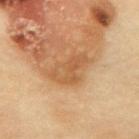This lesion was catalogued during total-body skin photography and was not selected for biopsy. On the upper back. A female subject aged 58 to 62. A 15 mm close-up extracted from a 3D total-body photography capture. Automated tile analysis of the lesion measured a footprint of about 11 mm², a shape eccentricity near 0.8, and a symmetry-axis asymmetry near 0.4. And it measured a within-lesion color-variation index near 3.5/10 and radial color variation of about 1. The software also gave an automated nevus-likeness rating near 0 out of 100.